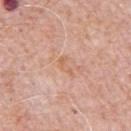Impression:
Part of a total-body skin-imaging series; this lesion was reviewed on a skin check and was not flagged for biopsy.
Context:
A region of skin cropped from a whole-body photographic capture, roughly 15 mm wide. Located on the chest. The recorded lesion diameter is about 2.5 mm. A male subject aged approximately 80.